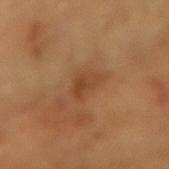<case>
<biopsy_status>not biopsied; imaged during a skin examination</biopsy_status>
<patient>
  <sex>female</sex>
  <age_approx>55</age_approx>
</patient>
<image>
  <source>total-body photography crop</source>
  <field_of_view_mm>15</field_of_view_mm>
</image>
<lesion_size>
  <long_diameter_mm_approx>3.0</long_diameter_mm_approx>
</lesion_size>
<site>left forearm</site>
</case>